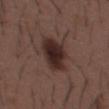workup: no biopsy performed (imaged during a skin exam) | patient: male, about 50 years old | anatomic site: the mid back | imaging modality: 15 mm crop, total-body photography | automated metrics: a lesion color around L≈27 a*≈16 b*≈19 in CIELAB, about 12 CIELAB-L* units darker than the surrounding skin, and a normalized lesion–skin contrast near 12; border irregularity of about 2 on a 0–10 scale, a within-lesion color-variation index near 4.5/10, and a peripheral color-asymmetry measure near 1; an automated nevus-likeness rating near 95 out of 100 | lesion size: ≈5.5 mm.